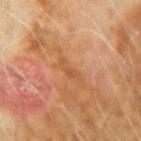workup: no biopsy performed (imaged during a skin exam)
image: 15 mm crop, total-body photography
illumination: cross-polarized
body site: the left upper arm
patient: male, roughly 70 years of age
lesion diameter: ≈3.5 mm
image-analysis metrics: a lesion area of about 3 mm², an eccentricity of roughly 0.95, and two-axis asymmetry of about 0.45; a mean CIELAB color near L≈44 a*≈21 b*≈34, a lesion–skin lightness drop of about 5, and a normalized border contrast of about 5; a classifier nevus-likeness of about 0/100 and a lesion-detection confidence of about 95/100